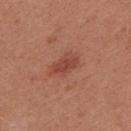Imaged during a routine full-body skin examination; the lesion was not biopsied and no histopathology is available. Cropped from a whole-body photographic skin survey; the tile spans about 15 mm. From the upper back. A female subject, aged 23 to 27. An algorithmic analysis of the crop reported a mean CIELAB color near L≈45 a*≈28 b*≈29, roughly 9 lightness units darker than nearby skin, and a lesion-to-skin contrast of about 7 (normalized; higher = more distinct). The analysis additionally found a color-variation rating of about 1.5/10 and a peripheral color-asymmetry measure near 0.5. Imaged with white-light lighting.